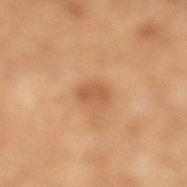No biopsy was performed on this lesion — it was imaged during a full skin examination and was not determined to be concerning. The tile uses cross-polarized illumination. A male subject, aged approximately 65. The lesion is on the left lower leg. A region of skin cropped from a whole-body photographic capture, roughly 15 mm wide. Approximately 3 mm at its widest. The lesion-visualizer software estimated a mean CIELAB color near L≈56 a*≈23 b*≈38, roughly 9 lightness units darker than nearby skin, and a normalized lesion–skin contrast near 6. The analysis additionally found a classifier nevus-likeness of about 5/100 and a detector confidence of about 100 out of 100 that the crop contains a lesion.